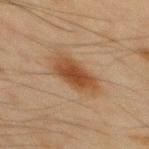{"biopsy_status": "not biopsied; imaged during a skin examination", "automated_metrics": {"area_mm2_approx": 14.0, "eccentricity": 0.85, "shape_asymmetry": 0.15, "cielab_L": 39, "cielab_a": 17, "cielab_b": 30, "vs_skin_darker_L": 9.0, "vs_skin_contrast_norm": 8.5, "color_variation_0_10": 4.0, "peripheral_color_asymmetry": 1.0, "nevus_likeness_0_100": 100, "lesion_detection_confidence_0_100": 100}, "lesion_size": {"long_diameter_mm_approx": 5.5}, "image": {"source": "total-body photography crop", "field_of_view_mm": 15}, "site": "back", "patient": {"sex": "male", "age_approx": 45}, "lighting": "cross-polarized"}